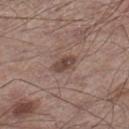Notes:
• follow-up — no biopsy performed (imaged during a skin exam)
• patient — male, aged around 55
• anatomic site — the right lower leg
• imaging modality — ~15 mm tile from a whole-body skin photo
• tile lighting — white-light
• size — ~3 mm (longest diameter)